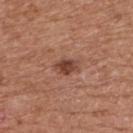No biopsy was performed on this lesion — it was imaged during a full skin examination and was not determined to be concerning. A male patient approximately 65 years of age. The lesion's longest dimension is about 3 mm. The total-body-photography lesion software estimated an area of roughly 4 mm², an outline eccentricity of about 0.75 (0 = round, 1 = elongated), and a symmetry-axis asymmetry near 0.25. It also reported a border-irregularity rating of about 2.5/10, internal color variation of about 2.5 on a 0–10 scale, and peripheral color asymmetry of about 1. The analysis additionally found an automated nevus-likeness rating near 80 out of 100 and a lesion-detection confidence of about 100/100. The tile uses white-light illumination. A 15 mm close-up extracted from a 3D total-body photography capture. The lesion is on the back.